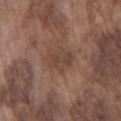Q: Was this lesion biopsied?
A: imaged on a skin check; not biopsied
Q: Who is the patient?
A: male, aged 73 to 77
Q: What is the imaging modality?
A: 15 mm crop, total-body photography
Q: What is the lesion's diameter?
A: about 3 mm
Q: What lighting was used for the tile?
A: white-light illumination
Q: What is the anatomic site?
A: the chest
Q: Automated lesion metrics?
A: a lesion area of about 5 mm², a shape eccentricity near 0.75, and a shape-asymmetry score of about 0.45 (0 = symmetric); a mean CIELAB color near L≈42 a*≈18 b*≈24, roughly 7 lightness units darker than nearby skin, and a normalized lesion–skin contrast near 6; an automated nevus-likeness rating near 0 out of 100 and a lesion-detection confidence of about 100/100